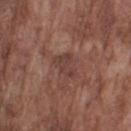follow-up = imaged on a skin check; not biopsied | automated lesion analysis = a classifier nevus-likeness of about 0/100 and lesion-presence confidence of about 95/100 | anatomic site = the arm | illumination = white-light illumination | patient = male, roughly 75 years of age | image source = 15 mm crop, total-body photography | diameter = ≈3.5 mm.